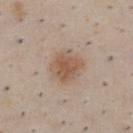Recorded during total-body skin imaging; not selected for excision or biopsy. Cropped from a total-body skin-imaging series; the visible field is about 15 mm. The lesion is located on the chest. The recorded lesion diameter is about 4 mm. A male patient, aged around 50.